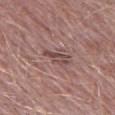Context: The total-body-photography lesion software estimated a nevus-likeness score of about 0/100 and a lesion-detection confidence of about 90/100. This image is a 15 mm lesion crop taken from a total-body photograph. The lesion is located on the leg. A male subject, aged 48 to 52.